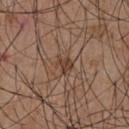Notes:
* diameter: ~2.5 mm (longest diameter)
* location: the chest
* automated metrics: an area of roughly 3.5 mm² and a symmetry-axis asymmetry near 0.3; a lesion–skin lightness drop of about 9 and a lesion-to-skin contrast of about 7.5 (normalized; higher = more distinct); a nevus-likeness score of about 0/100
* tile lighting: white-light
* acquisition: ~15 mm crop, total-body skin-cancer survey
* patient: male, aged around 55